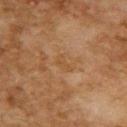The lesion was tiled from a total-body skin photograph and was not biopsied. A female patient, roughly 60 years of age. Automated image analysis of the tile measured a lesion color around L≈44 a*≈18 b*≈33 in CIELAB, about 4 CIELAB-L* units darker than the surrounding skin, and a normalized border contrast of about 4.5. It also reported radial color variation of about 0. A roughly 15 mm field-of-view crop from a total-body skin photograph. From the upper back. The tile uses cross-polarized illumination.